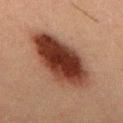Imaged during a routine full-body skin examination; the lesion was not biopsied and no histopathology is available.
About 8.5 mm across.
The tile uses cross-polarized illumination.
A male subject, aged 48–52.
The lesion is located on the upper back.
Automated tile analysis of the lesion measured an eccentricity of roughly 0.85 and a shape-asymmetry score of about 0.15 (0 = symmetric). And it measured a lesion color around L≈30 a*≈19 b*≈23 in CIELAB, about 15 CIELAB-L* units darker than the surrounding skin, and a normalized lesion–skin contrast near 14. The software also gave a classifier nevus-likeness of about 100/100 and a lesion-detection confidence of about 100/100.
Cropped from a total-body skin-imaging series; the visible field is about 15 mm.